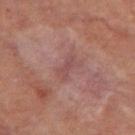{
  "image": {
    "source": "total-body photography crop",
    "field_of_view_mm": 15
  },
  "site": "left thigh",
  "patient": {
    "sex": "male",
    "age_approx": 60
  },
  "automated_metrics": {
    "cielab_L": 46,
    "cielab_a": 22,
    "cielab_b": 21,
    "vs_skin_darker_L": 6.0,
    "vs_skin_contrast_norm": 5.0,
    "border_irregularity_0_10": 4.5,
    "lesion_detection_confidence_0_100": 95
  },
  "lighting": "cross-polarized",
  "lesion_size": {
    "long_diameter_mm_approx": 3.0
  }
}